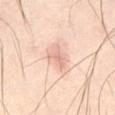Recorded during total-body skin imaging; not selected for excision or biopsy.
From the abdomen.
The tile uses cross-polarized illumination.
The subject is a male aged approximately 55.
Automated image analysis of the tile measured a footprint of about 4.5 mm², an eccentricity of roughly 0.8, and a symmetry-axis asymmetry near 0.4. The software also gave a lesion color around L≈74 a*≈21 b*≈29 in CIELAB, roughly 9 lightness units darker than nearby skin, and a normalized lesion–skin contrast near 5.5. And it measured an automated nevus-likeness rating near 0 out of 100 and a lesion-detection confidence of about 100/100.
A 15 mm crop from a total-body photograph taken for skin-cancer surveillance.